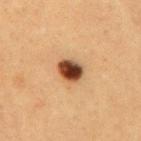Part of a total-body skin-imaging series; this lesion was reviewed on a skin check and was not flagged for biopsy. The patient is a female aged around 40. The lesion's longest dimension is about 3 mm. On the mid back. The tile uses cross-polarized illumination. Cropped from a total-body skin-imaging series; the visible field is about 15 mm.